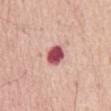Assessment:
No biopsy was performed on this lesion — it was imaged during a full skin examination and was not determined to be concerning.
Image and clinical context:
A region of skin cropped from a whole-body photographic capture, roughly 15 mm wide. The subject is a male in their mid-50s. From the front of the torso. The total-body-photography lesion software estimated a lesion color around L≈52 a*≈33 b*≈21 in CIELAB, a lesion–skin lightness drop of about 21, and a lesion-to-skin contrast of about 13.5 (normalized; higher = more distinct). And it measured a peripheral color-asymmetry measure near 1. Imaged with white-light lighting. About 2.5 mm across.